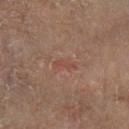Assessment:
Captured during whole-body skin photography for melanoma surveillance; the lesion was not biopsied.
Image and clinical context:
A male patient, in their 70s. This image is a 15 mm lesion crop taken from a total-body photograph. The lesion is located on the leg.